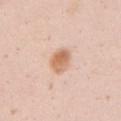The lesion was tiled from a total-body skin photograph and was not biopsied. Approximately 4 mm at its widest. A 15 mm close-up extracted from a 3D total-body photography capture. The total-body-photography lesion software estimated a border-irregularity rating of about 2/10, a within-lesion color-variation index near 4/10, and peripheral color asymmetry of about 1.5. The tile uses white-light illumination. The lesion is located on the left upper arm. The patient is a female aged approximately 20.